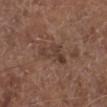biopsy_status: not biopsied; imaged during a skin examination
patient:
  sex: male
  age_approx: 75
site: left lower leg
lesion_size:
  long_diameter_mm_approx: 4.5
image:
  source: total-body photography crop
  field_of_view_mm: 15
lighting: white-light
automated_metrics:
  area_mm2_approx: 6.5
  eccentricity: 0.9
  border_irregularity_0_10: 6.0
  color_variation_0_10: 5.0
  peripheral_color_asymmetry: 2.0
  lesion_detection_confidence_0_100: 100